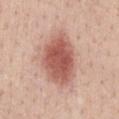Clinical impression: Imaged during a routine full-body skin examination; the lesion was not biopsied and no histopathology is available. Clinical summary: A 15 mm close-up extracted from a 3D total-body photography capture. Automated tile analysis of the lesion measured an average lesion color of about L≈56 a*≈25 b*≈27 (CIELAB), roughly 15 lightness units darker than nearby skin, and a normalized border contrast of about 9.5. And it measured a border-irregularity rating of about 2/10, a within-lesion color-variation index near 4.5/10, and a peripheral color-asymmetry measure near 1. Captured under white-light illumination. From the chest. The subject is a male approximately 40 years of age.